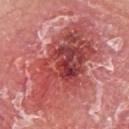Q: Was this lesion biopsied?
A: total-body-photography surveillance lesion; no biopsy
Q: Patient demographics?
A: male, in their mid- to late 60s
Q: What kind of image is this?
A: 15 mm crop, total-body photography
Q: How large is the lesion?
A: ≈12 mm
Q: What is the anatomic site?
A: the upper back
Q: What did automated image analysis measure?
A: a mean CIELAB color near L≈49 a*≈35 b*≈26, about 12 CIELAB-L* units darker than the surrounding skin, and a lesion-to-skin contrast of about 8.5 (normalized; higher = more distinct); an automated nevus-likeness rating near 5 out of 100 and a detector confidence of about 100 out of 100 that the crop contains a lesion
Q: How was the tile lit?
A: white-light